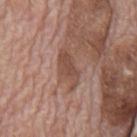Q: What is the lesion's diameter?
A: ≈4.5 mm
Q: Automated lesion metrics?
A: a mean CIELAB color near L≈48 a*≈20 b*≈26 and a normalized border contrast of about 7; border irregularity of about 3.5 on a 0–10 scale, a within-lesion color-variation index near 2/10, and peripheral color asymmetry of about 1; a nevus-likeness score of about 0/100 and lesion-presence confidence of about 100/100
Q: Illumination type?
A: white-light
Q: What is the imaging modality?
A: ~15 mm crop, total-body skin-cancer survey
Q: What are the patient's age and sex?
A: male, aged 68 to 72
Q: Lesion location?
A: the mid back